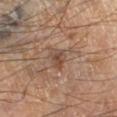Clinical impression:
Recorded during total-body skin imaging; not selected for excision or biopsy.
Background:
On the left lower leg. This is a cross-polarized tile. Approximately 2.5 mm at its widest. A male patient aged 68–72. This image is a 15 mm lesion crop taken from a total-body photograph. Automated tile analysis of the lesion measured a footprint of about 3.5 mm², an outline eccentricity of about 0.7 (0 = round, 1 = elongated), and a shape-asymmetry score of about 0.3 (0 = symmetric). And it measured a border-irregularity index near 2.5/10, a within-lesion color-variation index near 3/10, and a peripheral color-asymmetry measure near 1.